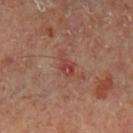The lesion was photographed on a routine skin check and not biopsied; there is no pathology result. The lesion is located on the leg. A male subject, aged around 65. A lesion tile, about 15 mm wide, cut from a 3D total-body photograph.